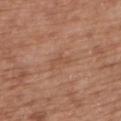The lesion was photographed on a routine skin check and not biopsied; there is no pathology result.
The total-body-photography lesion software estimated an area of roughly 2.5 mm² and a shape eccentricity near 0.9. And it measured a mean CIELAB color near L≈51 a*≈22 b*≈31, a lesion–skin lightness drop of about 6, and a lesion-to-skin contrast of about 4.5 (normalized; higher = more distinct).
The lesion's longest dimension is about 2.5 mm.
A lesion tile, about 15 mm wide, cut from a 3D total-body photograph.
Located on the upper back.
A male subject approximately 50 years of age.
Captured under white-light illumination.